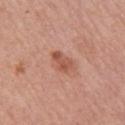  biopsy_status: not biopsied; imaged during a skin examination
  lighting: white-light
  automated_metrics:
    eccentricity: 0.85
    border_irregularity_0_10: 3.5
    color_variation_0_10: 2.5
    peripheral_color_asymmetry: 1.0
    lesion_detection_confidence_0_100: 100
  site: right upper arm
  image:
    source: total-body photography crop
    field_of_view_mm: 15
  lesion_size:
    long_diameter_mm_approx: 3.0
  patient:
    sex: female
    age_approx: 60